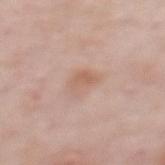<lesion>
  <biopsy_status>not biopsied; imaged during a skin examination</biopsy_status>
  <lesion_size>
    <long_diameter_mm_approx>3.0</long_diameter_mm_approx>
  </lesion_size>
  <patient>
    <sex>female</sex>
    <age_approx>50</age_approx>
  </patient>
  <site>upper back</site>
  <lighting>white-light</lighting>
  <automated_metrics>
    <vs_skin_contrast_norm>5.5</vs_skin_contrast_norm>
    <border_irregularity_0_10>3.0</border_irregularity_0_10>
    <color_variation_0_10>3.5</color_variation_0_10>
  </automated_metrics>
  <image>
    <source>total-body photography crop</source>
    <field_of_view_mm>15</field_of_view_mm>
  </image>
</lesion>